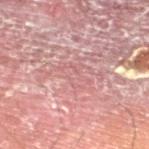Recorded during total-body skin imaging; not selected for excision or biopsy. This is a cross-polarized tile. This image is a 15 mm lesion crop taken from a total-body photograph. About 4 mm across. The lesion is on the left lower leg. A male subject, approximately 55 years of age.